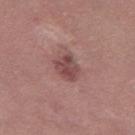workup: no biopsy performed (imaged during a skin exam); patient: female, in their mid-30s; location: the left thigh; image source: ~15 mm crop, total-body skin-cancer survey; illumination: white-light illumination; lesion size: about 3.5 mm.